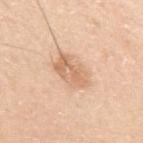| field | value |
|---|---|
| workup | catalogued during a skin exam; not biopsied |
| body site | the left upper arm |
| lesion size | about 4.5 mm |
| patient | male, approximately 30 years of age |
| acquisition | ~15 mm tile from a whole-body skin photo |
| illumination | white-light illumination |